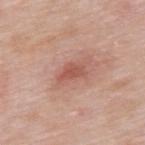{"biopsy_status": "not biopsied; imaged during a skin examination", "site": "mid back", "automated_metrics": {"border_irregularity_0_10": 3.5, "color_variation_0_10": 2.0, "peripheral_color_asymmetry": 0.5}, "lesion_size": {"long_diameter_mm_approx": 3.0}, "patient": {"sex": "male", "age_approx": 55}, "image": {"source": "total-body photography crop", "field_of_view_mm": 15}, "lighting": "white-light"}